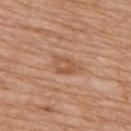Notes:
- biopsy status · imaged on a skin check; not biopsied
- anatomic site · the upper back
- illumination · white-light
- automated metrics · a footprint of about 5.5 mm², a shape eccentricity near 0.8, and a symmetry-axis asymmetry near 0.35; an average lesion color of about L≈54 a*≈22 b*≈34 (CIELAB), a lesion–skin lightness drop of about 8, and a normalized lesion–skin contrast near 6
- imaging modality · 15 mm crop, total-body photography
- subject · female, aged 73 to 77
- lesion size · ≈3.5 mm